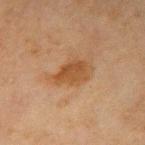notes = total-body-photography surveillance lesion; no biopsy.